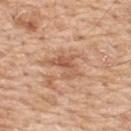Q: Who is the patient?
A: male, aged 58 to 62
Q: Where on the body is the lesion?
A: the upper back
Q: What lighting was used for the tile?
A: white-light
Q: Lesion size?
A: ~4 mm (longest diameter)
Q: How was this image acquired?
A: ~15 mm crop, total-body skin-cancer survey